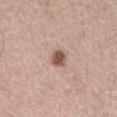| field | value |
|---|---|
| location | the abdomen |
| patient | male, aged 63–67 |
| acquisition | total-body-photography crop, ~15 mm field of view |
| image-analysis metrics | a shape eccentricity near 0.5 and two-axis asymmetry of about 0.25; a classifier nevus-likeness of about 100/100 and a lesion-detection confidence of about 100/100 |
| lesion diameter | about 2.5 mm |
| tile lighting | white-light |